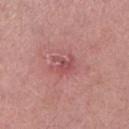No biopsy was performed on this lesion — it was imaged during a full skin examination and was not determined to be concerning.
A male patient, aged 38–42.
The total-body-photography lesion software estimated a mean CIELAB color near L≈51 a*≈29 b*≈22, roughly 8 lightness units darker than nearby skin, and a normalized border contrast of about 6.
From the right lower leg.
A close-up tile cropped from a whole-body skin photograph, about 15 mm across.
Captured under white-light illumination.